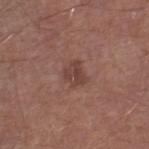No biopsy was performed on this lesion — it was imaged during a full skin examination and was not determined to be concerning. An algorithmic analysis of the crop reported border irregularity of about 3 on a 0–10 scale, a within-lesion color-variation index near 3/10, and a peripheral color-asymmetry measure near 1. The software also gave an automated nevus-likeness rating near 20 out of 100. The tile uses white-light illumination. A male subject in their mid- to late 50s. The lesion is located on the right lower leg. A 15 mm close-up tile from a total-body photography series done for melanoma screening. Longest diameter approximately 2.5 mm.